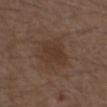follow-up: catalogued during a skin exam; not biopsied | automated lesion analysis: a footprint of about 9 mm², an eccentricity of roughly 0.7, and a shape-asymmetry score of about 0.25 (0 = symmetric); a border-irregularity rating of about 2.5/10 and a color-variation rating of about 2/10; a classifier nevus-likeness of about 15/100 and lesion-presence confidence of about 100/100 | image: ~15 mm crop, total-body skin-cancer survey | tile lighting: white-light illumination | body site: the chest | subject: male, aged 73–77.